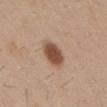Clinical impression: Part of a total-body skin-imaging series; this lesion was reviewed on a skin check and was not flagged for biopsy. Background: A close-up tile cropped from a whole-body skin photograph, about 15 mm across. Longest diameter approximately 3.5 mm. On the abdomen. The subject is a male aged approximately 30. Imaged with white-light lighting.